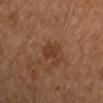Assessment:
Captured during whole-body skin photography for melanoma surveillance; the lesion was not biopsied.
Clinical summary:
Located on the left arm. A female patient, aged 38 to 42. Cropped from a whole-body photographic skin survey; the tile spans about 15 mm. The total-body-photography lesion software estimated a footprint of about 4 mm² and a symmetry-axis asymmetry near 0.2. And it measured radial color variation of about 0.5. The software also gave lesion-presence confidence of about 100/100. About 2.5 mm across.